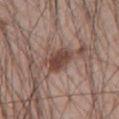The lesion was tiled from a total-body skin photograph and was not biopsied.